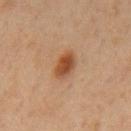Notes:
* notes — imaged on a skin check; not biopsied
* lighting — cross-polarized illumination
* acquisition — 15 mm crop, total-body photography
* size — ~3.5 mm (longest diameter)
* subject — male, approximately 60 years of age
* anatomic site — the mid back
* image-analysis metrics — an area of roughly 6 mm²